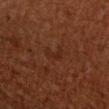Captured during whole-body skin photography for melanoma surveillance; the lesion was not biopsied.
A close-up tile cropped from a whole-body skin photograph, about 15 mm across.
The lesion is on the right upper arm.
A male patient about 60 years old.
Captured under cross-polarized illumination.